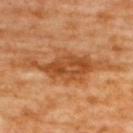Clinical impression: Imaged during a routine full-body skin examination; the lesion was not biopsied and no histopathology is available. Background: This is a cross-polarized tile. The lesion is on the back. Measured at roughly 12 mm in maximum diameter. This image is a 15 mm lesion crop taken from a total-body photograph. The subject is a female approximately 65 years of age.